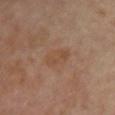tile lighting = cross-polarized | patient = female, about 40 years old | lesion diameter = ≈3.5 mm | body site = the front of the torso | image source = ~15 mm tile from a whole-body skin photo | TBP lesion metrics = a mean CIELAB color near L≈49 a*≈18 b*≈31 and a lesion–skin lightness drop of about 5; peripheral color asymmetry of about 0.5.